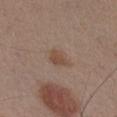Q: Is there a histopathology result?
A: total-body-photography surveillance lesion; no biopsy
Q: What kind of image is this?
A: ~15 mm crop, total-body skin-cancer survey
Q: Patient demographics?
A: male, about 55 years old
Q: Where on the body is the lesion?
A: the chest
Q: How large is the lesion?
A: about 2.5 mm
Q: How was the tile lit?
A: white-light illumination